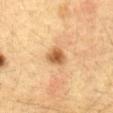follow-up = no biopsy performed (imaged during a skin exam)
patient = female, about 30 years old
location = the abdomen
imaging modality = ~15 mm crop, total-body skin-cancer survey